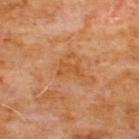Part of a total-body skin-imaging series; this lesion was reviewed on a skin check and was not flagged for biopsy. Measured at roughly 3 mm in maximum diameter. A 15 mm crop from a total-body photograph taken for skin-cancer surveillance. A male subject, in their 60s. On the front of the torso. The total-body-photography lesion software estimated an area of roughly 3.5 mm², an outline eccentricity of about 0.85 (0 = round, 1 = elongated), and a symmetry-axis asymmetry near 0.5. The software also gave a mean CIELAB color near L≈51 a*≈25 b*≈40, a lesion–skin lightness drop of about 6, and a normalized border contrast of about 5.5. The analysis additionally found a classifier nevus-likeness of about 0/100 and a detector confidence of about 100 out of 100 that the crop contains a lesion. The tile uses cross-polarized illumination.